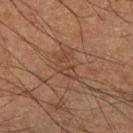Impression:
No biopsy was performed on this lesion — it was imaged during a full skin examination and was not determined to be concerning.
Acquisition and patient details:
A 15 mm close-up extracted from a 3D total-body photography capture. The tile uses cross-polarized illumination. Located on the right lower leg. The subject is a male aged 58 to 62. About 3.5 mm across.